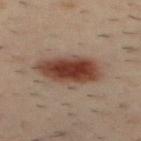Clinical impression: The lesion was tiled from a total-body skin photograph and was not biopsied. Clinical summary: A male subject aged 38–42. The lesion is on the upper back. This is a cross-polarized tile. A 15 mm close-up extracted from a 3D total-body photography capture.